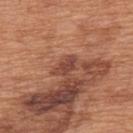The lesion was photographed on a routine skin check and not biopsied; there is no pathology result. About 3 mm across. On the upper back. A region of skin cropped from a whole-body photographic capture, roughly 15 mm wide. This is a white-light tile. The subject is a male in their mid- to late 60s.